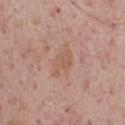Q: Was a biopsy performed?
A: total-body-photography surveillance lesion; no biopsy
Q: Where on the body is the lesion?
A: the chest
Q: How large is the lesion?
A: ~3.5 mm (longest diameter)
Q: What is the imaging modality?
A: total-body-photography crop, ~15 mm field of view
Q: Patient demographics?
A: male, aged around 75
Q: Automated lesion metrics?
A: a lesion color around L≈57 a*≈20 b*≈28 in CIELAB, a lesion–skin lightness drop of about 6, and a lesion-to-skin contrast of about 5 (normalized; higher = more distinct); a border-irregularity index near 4.5/10, a color-variation rating of about 1.5/10, and peripheral color asymmetry of about 0.5; an automated nevus-likeness rating near 0 out of 100 and lesion-presence confidence of about 100/100